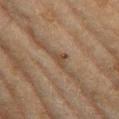This lesion was catalogued during total-body skin photography and was not selected for biopsy.
The total-body-photography lesion software estimated a classifier nevus-likeness of about 0/100 and lesion-presence confidence of about 50/100.
The lesion is located on the left thigh.
The subject is a female roughly 60 years of age.
The tile uses cross-polarized illumination.
A 15 mm close-up tile from a total-body photography series done for melanoma screening.